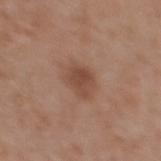{"biopsy_status": "not biopsied; imaged during a skin examination"}